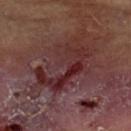Findings:
• notes — total-body-photography surveillance lesion; no biopsy
• lesion diameter — ~9.5 mm (longest diameter)
• tile lighting — cross-polarized illumination
• acquisition — total-body-photography crop, ~15 mm field of view
• subject — male, aged around 70
• anatomic site — the left lower leg